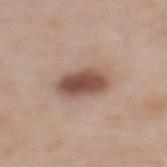Acquisition and patient details:
The total-body-photography lesion software estimated a lesion-detection confidence of about 100/100. The subject is a female aged approximately 65. A 15 mm close-up extracted from a 3D total-body photography capture. The lesion's longest dimension is about 5 mm. The tile uses white-light illumination. On the mid back.